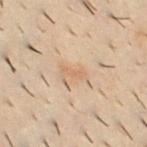Imaged during a routine full-body skin examination; the lesion was not biopsied and no histopathology is available. This image is a 15 mm lesion crop taken from a total-body photograph. On the front of the torso. A male patient aged 28–32. The recorded lesion diameter is about 2.5 mm. Automated tile analysis of the lesion measured a within-lesion color-variation index near 0/10 and peripheral color asymmetry of about 0. The software also gave a classifier nevus-likeness of about 0/100. This is a cross-polarized tile.